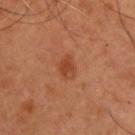follow-up — no biopsy performed (imaged during a skin exam); lesion diameter — ~3 mm (longest diameter); illumination — cross-polarized; patient — male, about 55 years old; location — the upper back; imaging modality — total-body-photography crop, ~15 mm field of view.